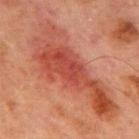Notes:
* notes · no biopsy performed (imaged during a skin exam)